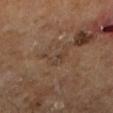A 15 mm close-up tile from a total-body photography series done for melanoma screening.
Approximately 3.5 mm at its widest.
A male patient, roughly 65 years of age.
On the leg.
The tile uses cross-polarized illumination.